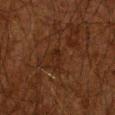Context: This image is a 15 mm lesion crop taken from a total-body photograph. The lesion is on the arm. The recorded lesion diameter is about 2.5 mm. This is a cross-polarized tile. The patient is a male aged around 60. Automated tile analysis of the lesion measured a footprint of about 2.5 mm² and a shape-asymmetry score of about 0.45 (0 = symmetric). And it measured an automated nevus-likeness rating near 0 out of 100 and a detector confidence of about 60 out of 100 that the crop contains a lesion.